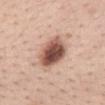Assessment: The lesion was photographed on a routine skin check and not biopsied; there is no pathology result. Acquisition and patient details: The lesion is located on the mid back. A region of skin cropped from a whole-body photographic capture, roughly 15 mm wide. About 4 mm across. Captured under white-light illumination. A female patient, in their mid-30s.